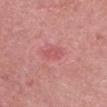Findings:
* workup — total-body-photography surveillance lesion; no biopsy
* subject — female, approximately 60 years of age
* location — the head or neck
* image source — ~15 mm crop, total-body skin-cancer survey